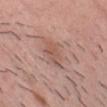Notes:
• follow-up — total-body-photography surveillance lesion; no biopsy
• automated lesion analysis — a nevus-likeness score of about 0/100 and lesion-presence confidence of about 100/100
• lesion diameter — ~3.5 mm (longest diameter)
• acquisition — 15 mm crop, total-body photography
• body site — the head or neck
• tile lighting — white-light
• patient — male, in their mid-20s